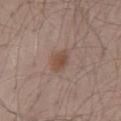Impression:
The lesion was tiled from a total-body skin photograph and was not biopsied.